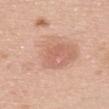Clinical impression: This lesion was catalogued during total-body skin photography and was not selected for biopsy. Context: Located on the upper back. The patient is a female aged 58 to 62. A lesion tile, about 15 mm wide, cut from a 3D total-body photograph.